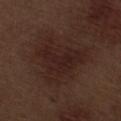A male subject aged approximately 70.
Imaged with white-light lighting.
About 6.5 mm across.
Cropped from a total-body skin-imaging series; the visible field is about 15 mm.
Automated image analysis of the tile measured a lesion area of about 22 mm², an eccentricity of roughly 0.5, and a symmetry-axis asymmetry near 0.5. It also reported a mean CIELAB color near L≈22 a*≈18 b*≈19. The software also gave a border-irregularity rating of about 6/10, a color-variation rating of about 3/10, and radial color variation of about 1.
On the leg.